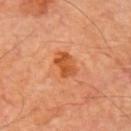Clinical impression:
The lesion was tiled from a total-body skin photograph and was not biopsied.
Context:
A region of skin cropped from a whole-body photographic capture, roughly 15 mm wide. The subject is a male aged approximately 60. On the arm.